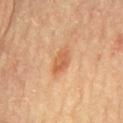A male subject in their mid-60s. A close-up tile cropped from a whole-body skin photograph, about 15 mm across. On the front of the torso. The tile uses cross-polarized illumination. The lesion-visualizer software estimated a lesion area of about 5.5 mm² and a shape eccentricity near 0.85. And it measured an average lesion color of about L≈59 a*≈25 b*≈39 (CIELAB) and a normalized lesion–skin contrast near 6.5. It also reported a border-irregularity index near 2/10, a color-variation rating of about 3/10, and a peripheral color-asymmetry measure near 1. And it measured an automated nevus-likeness rating near 85 out of 100. Measured at roughly 3.5 mm in maximum diameter.